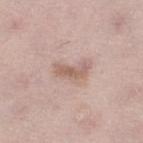Recorded during total-body skin imaging; not selected for excision or biopsy. An algorithmic analysis of the crop reported a nevus-likeness score of about 20/100 and a detector confidence of about 100 out of 100 that the crop contains a lesion. Located on the leg. A close-up tile cropped from a whole-body skin photograph, about 15 mm across. Approximately 4 mm at its widest. This is a white-light tile. A female subject aged around 50.